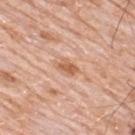Clinical impression:
Recorded during total-body skin imaging; not selected for excision or biopsy.